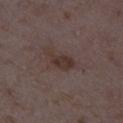Findings:
• biopsy status · no biopsy performed (imaged during a skin exam)
• lesion diameter · about 4 mm
• image source · total-body-photography crop, ~15 mm field of view
• anatomic site · the left lower leg
• automated metrics · an eccentricity of roughly 0.85 and two-axis asymmetry of about 0.4; border irregularity of about 4.5 on a 0–10 scale, a within-lesion color-variation index near 2/10, and a peripheral color-asymmetry measure near 0.5; an automated nevus-likeness rating near 10 out of 100 and lesion-presence confidence of about 100/100
• tile lighting · white-light illumination
• patient · female, in their mid-30s